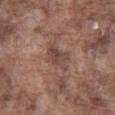Captured during whole-body skin photography for melanoma surveillance; the lesion was not biopsied.
The recorded lesion diameter is about 4 mm.
From the front of the torso.
This image is a 15 mm lesion crop taken from a total-body photograph.
This is a white-light tile.
A male subject, aged approximately 75.